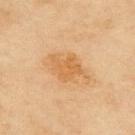Q: Was a biopsy performed?
A: total-body-photography surveillance lesion; no biopsy
Q: How was this image acquired?
A: ~15 mm tile from a whole-body skin photo
Q: Patient demographics?
A: female, aged 58–62
Q: What lighting was used for the tile?
A: cross-polarized
Q: Automated lesion metrics?
A: an area of roughly 9 mm² and an outline eccentricity of about 0.7 (0 = round, 1 = elongated); an automated nevus-likeness rating near 35 out of 100
Q: How large is the lesion?
A: ≈4.5 mm
Q: Lesion location?
A: the upper back